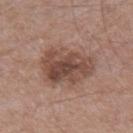No biopsy was performed on this lesion — it was imaged during a full skin examination and was not determined to be concerning. A male patient, roughly 65 years of age. A 15 mm close-up tile from a total-body photography series done for melanoma screening. Captured under white-light illumination. Measured at roughly 6 mm in maximum diameter. On the right thigh. An algorithmic analysis of the crop reported a mean CIELAB color near L≈47 a*≈19 b*≈25, a lesion–skin lightness drop of about 11, and a lesion-to-skin contrast of about 8.5 (normalized; higher = more distinct).